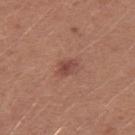Clinical impression: Captured during whole-body skin photography for melanoma surveillance; the lesion was not biopsied. Background: The recorded lesion diameter is about 2.5 mm. The lesion is on the arm. This image is a 15 mm lesion crop taken from a total-body photograph. The lesion-visualizer software estimated a lesion area of about 3.5 mm², an eccentricity of roughly 0.7, and a shape-asymmetry score of about 0.3 (0 = symmetric). And it measured an average lesion color of about L≈46 a*≈24 b*≈26 (CIELAB) and a lesion–skin lightness drop of about 9. The software also gave a border-irregularity index near 2.5/10, a color-variation rating of about 3/10, and peripheral color asymmetry of about 1. The subject is a male aged around 25.